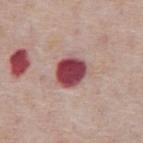Findings:
• follow-up · catalogued during a skin exam; not biopsied
• patient · male, aged approximately 75
• lesion size · ~3.5 mm (longest diameter)
• tile lighting · white-light
• anatomic site · the abdomen
• image · total-body-photography crop, ~15 mm field of view